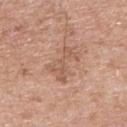Q: Was this lesion biopsied?
A: total-body-photography surveillance lesion; no biopsy
Q: What lighting was used for the tile?
A: white-light
Q: How large is the lesion?
A: ~4.5 mm (longest diameter)
Q: What is the imaging modality?
A: ~15 mm crop, total-body skin-cancer survey
Q: What is the anatomic site?
A: the back
Q: Who is the patient?
A: male, aged 53 to 57